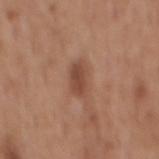{"biopsy_status": "not biopsied; imaged during a skin examination", "image": {"source": "total-body photography crop", "field_of_view_mm": 15}, "patient": {"sex": "male", "age_approx": 60}, "lesion_size": {"long_diameter_mm_approx": 3.5}, "site": "mid back"}